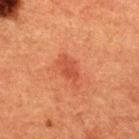Q: What is the imaging modality?
A: ~15 mm tile from a whole-body skin photo
Q: Lesion location?
A: the upper back
Q: Patient demographics?
A: male, about 50 years old
Q: Automated lesion metrics?
A: an area of roughly 4 mm² and two-axis asymmetry of about 0.3; an average lesion color of about L≈41 a*≈29 b*≈32 (CIELAB) and a normalized lesion–skin contrast near 5.5; a border-irregularity rating of about 3/10 and a within-lesion color-variation index near 1.5/10
Q: How was the tile lit?
A: cross-polarized illumination
Q: Lesion size?
A: about 3 mm